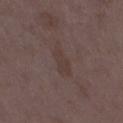<lesion>
<biopsy_status>not biopsied; imaged during a skin examination</biopsy_status>
<patient>
  <sex>female</sex>
  <age_approx>35</age_approx>
</patient>
<image>
  <source>total-body photography crop</source>
  <field_of_view_mm>15</field_of_view_mm>
</image>
<site>right thigh</site>
</lesion>